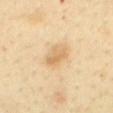Acquisition and patient details:
On the mid back. A female patient about 40 years old. Captured under cross-polarized illumination. The recorded lesion diameter is about 3.5 mm. The total-body-photography lesion software estimated a footprint of about 5 mm², a shape eccentricity near 0.85, and a shape-asymmetry score of about 0.3 (0 = symmetric). The software also gave a mean CIELAB color near L≈71 a*≈17 b*≈42, roughly 9 lightness units darker than nearby skin, and a lesion-to-skin contrast of about 6 (normalized; higher = more distinct). And it measured border irregularity of about 3 on a 0–10 scale, a color-variation rating of about 3.5/10, and radial color variation of about 1. Cropped from a total-body skin-imaging series; the visible field is about 15 mm.